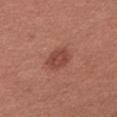Q: Was a biopsy performed?
A: no biopsy performed (imaged during a skin exam)
Q: What are the patient's age and sex?
A: female, in their mid- to late 30s
Q: How was this image acquired?
A: ~15 mm tile from a whole-body skin photo
Q: Lesion location?
A: the left upper arm